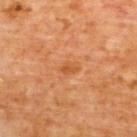Q: Was this lesion biopsied?
A: imaged on a skin check; not biopsied
Q: Patient demographics?
A: male, aged 58 to 62
Q: What did automated image analysis measure?
A: an area of roughly 2.5 mm² and two-axis asymmetry of about 0.25; a mean CIELAB color near L≈53 a*≈27 b*≈43 and about 7 CIELAB-L* units darker than the surrounding skin
Q: Lesion location?
A: the upper back
Q: How large is the lesion?
A: ≈2 mm
Q: What is the imaging modality?
A: ~15 mm crop, total-body skin-cancer survey